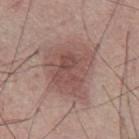{"biopsy_status": "not biopsied; imaged during a skin examination", "lesion_size": {"long_diameter_mm_approx": 7.0}, "site": "abdomen", "image": {"source": "total-body photography crop", "field_of_view_mm": 15}, "lighting": "white-light", "patient": {"sex": "male", "age_approx": 55}}